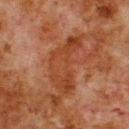Impression:
Part of a total-body skin-imaging series; this lesion was reviewed on a skin check and was not flagged for biopsy.
Acquisition and patient details:
A 15 mm crop from a total-body photograph taken for skin-cancer surveillance. This is a cross-polarized tile. Automated tile analysis of the lesion measured an average lesion color of about L≈33 a*≈22 b*≈30 (CIELAB) and a normalized lesion–skin contrast near 6.5. It also reported a border-irregularity index near 7/10, a color-variation rating of about 3.5/10, and radial color variation of about 1. And it measured lesion-presence confidence of about 95/100. From the upper back. A male subject roughly 80 years of age. Measured at roughly 7.5 mm in maximum diameter.